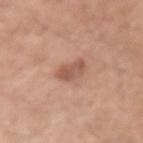<tbp_lesion>
<biopsy_status>not biopsied; imaged during a skin examination</biopsy_status>
<patient>
  <sex>male</sex>
  <age_approx>55</age_approx>
</patient>
<lighting>white-light</lighting>
<lesion_size>
  <long_diameter_mm_approx>3.0</long_diameter_mm_approx>
</lesion_size>
<image>
  <source>total-body photography crop</source>
  <field_of_view_mm>15</field_of_view_mm>
</image>
<site>left upper arm</site>
</tbp_lesion>